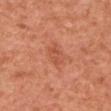| key | value |
|---|---|
| workup | imaged on a skin check; not biopsied |
| image source | ~15 mm crop, total-body skin-cancer survey |
| subject | female, in their 50s |
| anatomic site | the right upper arm |
| size | ~2.5 mm (longest diameter) |
| tile lighting | cross-polarized illumination |